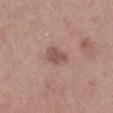| key | value |
|---|---|
| follow-up | total-body-photography surveillance lesion; no biopsy |
| patient | female, aged around 60 |
| lighting | white-light illumination |
| site | the leg |
| image | 15 mm crop, total-body photography |
| size | ~3 mm (longest diameter) |